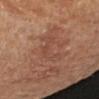biopsy status = no biopsy performed (imaged during a skin exam); site = the left arm; acquisition = 15 mm crop, total-body photography; tile lighting = cross-polarized; subject = female, in their mid-60s.